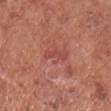biopsy_status: not biopsied; imaged during a skin examination
patient:
  sex: male
  age_approx: 75
site: front of the torso
image:
  source: total-body photography crop
  field_of_view_mm: 15